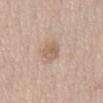{
  "biopsy_status": "not biopsied; imaged during a skin examination",
  "patient": {
    "sex": "male",
    "age_approx": 60
  },
  "lighting": "white-light",
  "image": {
    "source": "total-body photography crop",
    "field_of_view_mm": 15
  },
  "automated_metrics": {
    "color_variation_0_10": 2.0,
    "peripheral_color_asymmetry": 0.5,
    "nevus_likeness_0_100": 15
  },
  "lesion_size": {
    "long_diameter_mm_approx": 2.5
  },
  "site": "front of the torso"
}